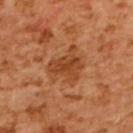| feature | finding |
|---|---|
| follow-up | catalogued during a skin exam; not biopsied |
| patient | female, aged 53–57 |
| automated lesion analysis | border irregularity of about 5 on a 0–10 scale and radial color variation of about 1 |
| image | ~15 mm crop, total-body skin-cancer survey |
| tile lighting | cross-polarized illumination |
| location | the upper back |
| lesion diameter | about 4.5 mm |